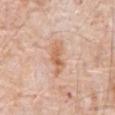<lesion>
<biopsy_status>not biopsied; imaged during a skin examination</biopsy_status>
<automated_metrics>
  <eccentricity>0.9</eccentricity>
  <shape_asymmetry>0.35</shape_asymmetry>
  <cielab_L>64</cielab_L>
  <cielab_a>22</cielab_a>
  <cielab_b>34</cielab_b>
  <vs_skin_darker_L>9.0</vs_skin_darker_L>
  <vs_skin_contrast_norm>7.5</vs_skin_contrast_norm>
  <border_irregularity_0_10>4.5</border_irregularity_0_10>
  <color_variation_0_10>3.5</color_variation_0_10>
  <peripheral_color_asymmetry>1.0</peripheral_color_asymmetry>
  <lesion_detection_confidence_0_100>100</lesion_detection_confidence_0_100>
</automated_metrics>
<lesion_size>
  <long_diameter_mm_approx>4.0</long_diameter_mm_approx>
</lesion_size>
<image>
  <source>total-body photography crop</source>
  <field_of_view_mm>15</field_of_view_mm>
</image>
<site>abdomen</site>
<patient>
  <sex>male</sex>
  <age_approx>80</age_approx>
</patient>
<lighting>white-light</lighting>
</lesion>